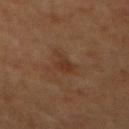anatomic site — the mid back | acquisition — total-body-photography crop, ~15 mm field of view | lighting — cross-polarized | patient — male, aged 58–62 | automated metrics — an eccentricity of roughly 0.7 and a symmetry-axis asymmetry near 0.35; a mean CIELAB color near L≈30 a*≈17 b*≈27 and about 6 CIELAB-L* units darker than the surrounding skin | diameter — ≈3 mm.